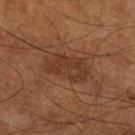The lesion was tiled from a total-body skin photograph and was not biopsied. The lesion's longest dimension is about 5.5 mm. A male subject, approximately 65 years of age. A region of skin cropped from a whole-body photographic capture, roughly 15 mm wide. The total-body-photography lesion software estimated a mean CIELAB color near L≈34 a*≈21 b*≈29, a lesion–skin lightness drop of about 7, and a lesion-to-skin contrast of about 6.5 (normalized; higher = more distinct). The software also gave a classifier nevus-likeness of about 15/100 and lesion-presence confidence of about 100/100. Captured under cross-polarized illumination. From the left leg.